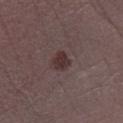Q: Was this lesion biopsied?
A: catalogued during a skin exam; not biopsied
Q: What kind of image is this?
A: 15 mm crop, total-body photography
Q: Who is the patient?
A: male, aged approximately 40
Q: Lesion location?
A: the right lower leg